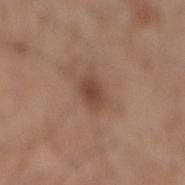Clinical impression: Part of a total-body skin-imaging series; this lesion was reviewed on a skin check and was not flagged for biopsy. Background: Located on the mid back. A male patient, aged 58–62. The total-body-photography lesion software estimated a color-variation rating of about 2/10 and radial color variation of about 0.5. And it measured an automated nevus-likeness rating near 70 out of 100 and lesion-presence confidence of about 100/100. Captured under white-light illumination. This image is a 15 mm lesion crop taken from a total-body photograph.